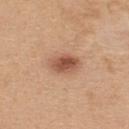The lesion was tiled from a total-body skin photograph and was not biopsied.
This image is a 15 mm lesion crop taken from a total-body photograph.
Imaged with white-light lighting.
The lesion is on the upper back.
The subject is a female roughly 25 years of age.